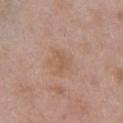patient: female, aged around 30 | location: the chest | image source: ~15 mm tile from a whole-body skin photo | size: ≈2.5 mm | illumination: white-light illumination.